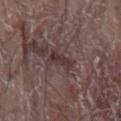follow-up: catalogued during a skin exam; not biopsied
subject: male, aged around 80
diameter: ~3 mm (longest diameter)
anatomic site: the right arm
acquisition: total-body-photography crop, ~15 mm field of view